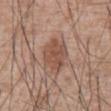Case summary:
– biopsy status: no biopsy performed (imaged during a skin exam)
– acquisition: ~15 mm crop, total-body skin-cancer survey
– automated lesion analysis: border irregularity of about 2 on a 0–10 scale, a within-lesion color-variation index near 3/10, and peripheral color asymmetry of about 1
– site: the abdomen
– patient: male, aged 58 to 62
– tile lighting: white-light